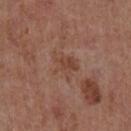Q: Is there a histopathology result?
A: imaged on a skin check; not biopsied
Q: Patient demographics?
A: male, aged 53 to 57
Q: Lesion location?
A: the chest
Q: What is the imaging modality?
A: ~15 mm crop, total-body skin-cancer survey